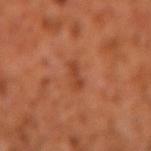{"biopsy_status": "not biopsied; imaged during a skin examination", "site": "arm", "lighting": "cross-polarized", "patient": {"sex": "male", "age_approx": 60}, "image": {"source": "total-body photography crop", "field_of_view_mm": 15}, "automated_metrics": {"border_irregularity_0_10": 3.0, "color_variation_0_10": 1.0, "peripheral_color_asymmetry": 0.5}}